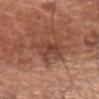workup = catalogued during a skin exam; not biopsied | image source = 15 mm crop, total-body photography | illumination = white-light illumination | body site = the front of the torso | diameter = ~5.5 mm (longest diameter) | patient = female, roughly 75 years of age.